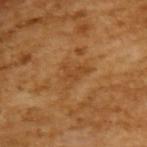This lesion was catalogued during total-body skin photography and was not selected for biopsy.
About 3.5 mm across.
A lesion tile, about 15 mm wide, cut from a 3D total-body photograph.
This is a cross-polarized tile.
A male subject aged approximately 65.
Automated tile analysis of the lesion measured a footprint of about 5.5 mm², a shape eccentricity near 0.75, and a shape-asymmetry score of about 0.55 (0 = symmetric). The software also gave about 6 CIELAB-L* units darker than the surrounding skin and a normalized lesion–skin contrast near 5. It also reported border irregularity of about 5.5 on a 0–10 scale, a color-variation rating of about 2/10, and a peripheral color-asymmetry measure near 0.5. It also reported an automated nevus-likeness rating near 0 out of 100 and a lesion-detection confidence of about 95/100.